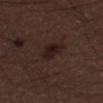Imaged with white-light lighting. The lesion is on the left thigh. A 15 mm crop from a total-body photograph taken for skin-cancer surveillance. Measured at roughly 3.5 mm in maximum diameter. The subject is a male aged 28 to 32. The lesion-visualizer software estimated a lesion color around L≈19 a*≈14 b*≈16 in CIELAB, a lesion–skin lightness drop of about 7, and a normalized lesion–skin contrast near 9.